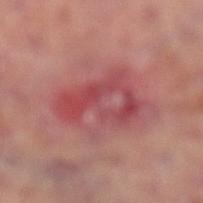The lesion is located on the left lower leg.
A male patient, about 70 years old.
A lesion tile, about 15 mm wide, cut from a 3D total-body photograph.